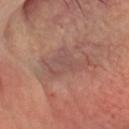biopsy status = imaged on a skin check; not biopsied | subject = male, in their mid-60s | location = the head or neck | acquisition = ~15 mm tile from a whole-body skin photo.